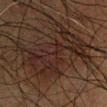Part of a total-body skin-imaging series; this lesion was reviewed on a skin check and was not flagged for biopsy. The patient is a male roughly 60 years of age. Located on the left upper arm. Captured under cross-polarized illumination. A 15 mm close-up tile from a total-body photography series done for melanoma screening. Automated image analysis of the tile measured a lesion color around L≈18 a*≈13 b*≈17 in CIELAB. It also reported a nevus-likeness score of about 0/100 and lesion-presence confidence of about 80/100. The recorded lesion diameter is about 10.5 mm.